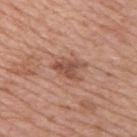biopsy status — total-body-photography surveillance lesion; no biopsy | lesion diameter — ≈3.5 mm | body site — the right upper arm | image — total-body-photography crop, ~15 mm field of view | lighting — white-light illumination | patient — female, roughly 45 years of age.